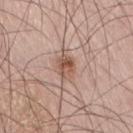Notes:
– follow-up — no biopsy performed (imaged during a skin exam)
– patient — male, aged approximately 60
– lesion diameter — ~3 mm (longest diameter)
– body site — the leg
– illumination — white-light
– image-analysis metrics — a footprint of about 5 mm², a shape eccentricity near 0.65, and two-axis asymmetry of about 0.15; roughly 11 lightness units darker than nearby skin and a normalized lesion–skin contrast near 7.5; border irregularity of about 1.5 on a 0–10 scale, a within-lesion color-variation index near 7/10, and peripheral color asymmetry of about 2.5
– imaging modality — 15 mm crop, total-body photography